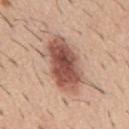Q: Was a biopsy performed?
A: no biopsy performed (imaged during a skin exam)
Q: Illumination type?
A: white-light illumination
Q: What is the imaging modality?
A: ~15 mm tile from a whole-body skin photo
Q: Lesion location?
A: the mid back
Q: Automated lesion metrics?
A: a footprint of about 24 mm² and an eccentricity of roughly 0.85; a lesion-to-skin contrast of about 10 (normalized; higher = more distinct)
Q: Lesion size?
A: ≈7.5 mm
Q: Patient demographics?
A: male, approximately 60 years of age